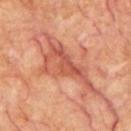  automated_metrics:
    area_mm2_approx: 20.0
    eccentricity: 0.9
    shape_asymmetry: 0.6
    cielab_L: 54
    cielab_a: 28
    cielab_b: 32
    vs_skin_darker_L: 10.0
    vs_skin_contrast_norm: 7.0
    nevus_likeness_0_100: 0
    lesion_detection_confidence_0_100: 85
  image:
    source: total-body photography crop
    field_of_view_mm: 15
  patient:
    sex: male
    age_approx: 65
  site: chest
  lighting: cross-polarized